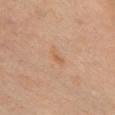Findings:
* notes · total-body-photography surveillance lesion; no biopsy
* site · the chest
* TBP lesion metrics · an area of roughly 2.5 mm²; an average lesion color of about L≈61 a*≈21 b*≈36 (CIELAB), a lesion–skin lightness drop of about 6, and a normalized border contrast of about 5.5; internal color variation of about 0 on a 0–10 scale; an automated nevus-likeness rating near 0 out of 100 and lesion-presence confidence of about 100/100
* subject · female, about 45 years old
* diameter · ~2.5 mm (longest diameter)
* acquisition · ~15 mm crop, total-body skin-cancer survey